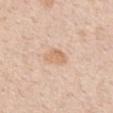{
  "lighting": "white-light",
  "patient": {
    "sex": "female",
    "age_approx": 45
  },
  "automated_metrics": {
    "area_mm2_approx": 4.5,
    "eccentricity": 0.85,
    "shape_asymmetry": 0.35,
    "nevus_likeness_0_100": 0,
    "lesion_detection_confidence_0_100": 100
  },
  "image": {
    "source": "total-body photography crop",
    "field_of_view_mm": 15
  },
  "lesion_size": {
    "long_diameter_mm_approx": 3.5
  },
  "site": "mid back"
}